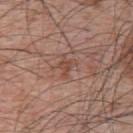The lesion was photographed on a routine skin check and not biopsied; there is no pathology result. The lesion is located on the upper back. A male patient, about 65 years old. A 15 mm crop from a total-body photograph taken for skin-cancer surveillance. The lesion's longest dimension is about 2.5 mm. This is a white-light tile. The total-body-photography lesion software estimated a lesion area of about 3.5 mm², a shape eccentricity near 0.75, and two-axis asymmetry of about 0.55. The software also gave a border-irregularity rating of about 6/10. The analysis additionally found a classifier nevus-likeness of about 0/100 and lesion-presence confidence of about 100/100.